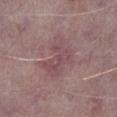Findings:
– notes · total-body-photography surveillance lesion; no biopsy
– subject · male, in their mid-70s
– acquisition · 15 mm crop, total-body photography
– body site · the leg
– lesion size · ≈5.5 mm
– lighting · white-light